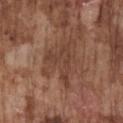Impression: No biopsy was performed on this lesion — it was imaged during a full skin examination and was not determined to be concerning. Background: Located on the chest. About 5.5 mm across. A 15 mm close-up extracted from a 3D total-body photography capture. A male patient, aged around 75.